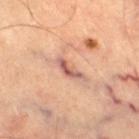Findings:
* notes: imaged on a skin check; not biopsied
* patient: male, roughly 60 years of age
* illumination: cross-polarized
* image: 15 mm crop, total-body photography
* site: the right thigh
* lesion size: about 4 mm